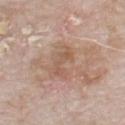Imaged during a routine full-body skin examination; the lesion was not biopsied and no histopathology is available. The lesion is located on the chest. A male patient aged around 80. A 15 mm close-up extracted from a 3D total-body photography capture. Automated tile analysis of the lesion measured a border-irregularity index near 7/10, a within-lesion color-variation index near 2/10, and peripheral color asymmetry of about 0. The recorded lesion diameter is about 3.5 mm.